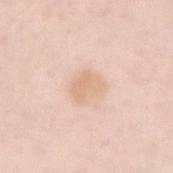| key | value |
|---|---|
| follow-up | catalogued during a skin exam; not biopsied |
| patient | female, aged approximately 65 |
| tile lighting | white-light |
| acquisition | 15 mm crop, total-body photography |
| lesion diameter | ~3.5 mm (longest diameter) |
| body site | the right forearm |